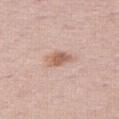Context:
The subject is a female aged 48–52. Captured under white-light illumination. A close-up tile cropped from a whole-body skin photograph, about 15 mm across. The recorded lesion diameter is about 3.5 mm. An algorithmic analysis of the crop reported a footprint of about 5.5 mm² and a shape-asymmetry score of about 0.2 (0 = symmetric). The analysis additionally found a mean CIELAB color near L≈61 a*≈20 b*≈28 and about 11 CIELAB-L* units darker than the surrounding skin. The software also gave a border-irregularity rating of about 2/10, internal color variation of about 5 on a 0–10 scale, and a peripheral color-asymmetry measure near 1.5. And it measured a nevus-likeness score of about 65/100 and a detector confidence of about 100 out of 100 that the crop contains a lesion. From the right thigh.